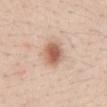Case summary:
* biopsy status · catalogued during a skin exam; not biopsied
* subject · female, roughly 25 years of age
* body site · the mid back
* acquisition · 15 mm crop, total-body photography
* diameter · ≈3 mm
* lighting · white-light illumination